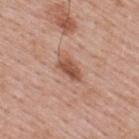A male subject, about 40 years old. A 15 mm close-up extracted from a 3D total-body photography capture. From the upper back.